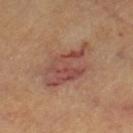image: 15 mm crop, total-body photography | subject: female, aged 58 to 62 | lighting: cross-polarized illumination | body site: the left thigh | TBP lesion metrics: a shape eccentricity near 0.85; roughly 9 lightness units darker than nearby skin and a lesion-to-skin contrast of about 7 (normalized; higher = more distinct); border irregularity of about 4.5 on a 0–10 scale and radial color variation of about 2; a detector confidence of about 100 out of 100 that the crop contains a lesion | lesion diameter: ≈7 mm.